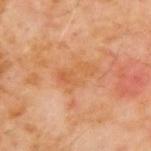– workup — total-body-photography surveillance lesion; no biopsy
– patient — male, aged 58–62
– site — the back
– diameter — about 4.5 mm
– acquisition — ~15 mm crop, total-body skin-cancer survey